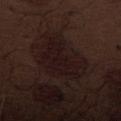Impression:
Part of a total-body skin-imaging series; this lesion was reviewed on a skin check and was not flagged for biopsy.
Clinical summary:
Captured under white-light illumination. A close-up tile cropped from a whole-body skin photograph, about 15 mm across. Automated tile analysis of the lesion measured an outline eccentricity of about 0.8 (0 = round, 1 = elongated) and a shape-asymmetry score of about 0.4 (0 = symmetric). It also reported a border-irregularity index near 5/10, a within-lesion color-variation index near 2.5/10, and peripheral color asymmetry of about 1. The software also gave an automated nevus-likeness rating near 0 out of 100 and a detector confidence of about 75 out of 100 that the crop contains a lesion. The lesion is located on the abdomen. Longest diameter approximately 7.5 mm. A male patient aged around 70.